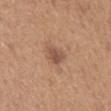Q: Is there a histopathology result?
A: imaged on a skin check; not biopsied
Q: What is the imaging modality?
A: ~15 mm crop, total-body skin-cancer survey
Q: Where on the body is the lesion?
A: the mid back
Q: How large is the lesion?
A: about 3 mm
Q: What are the patient's age and sex?
A: male, aged 63 to 67